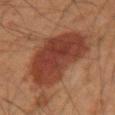<case>
<biopsy_status>not biopsied; imaged during a skin examination</biopsy_status>
<lighting>cross-polarized</lighting>
<patient>
  <sex>male</sex>
  <age_approx>50</age_approx>
</patient>
<automated_metrics>
  <eccentricity>0.55</eccentricity>
</automated_metrics>
<site>right upper arm</site>
<image>
  <source>total-body photography crop</source>
  <field_of_view_mm>15</field_of_view_mm>
</image>
</case>